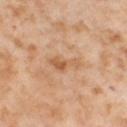notes: total-body-photography surveillance lesion; no biopsy | location: the leg | diameter: ~3.5 mm (longest diameter) | image: total-body-photography crop, ~15 mm field of view | patient: female, about 55 years old.